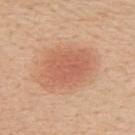Captured during whole-body skin photography for melanoma surveillance; the lesion was not biopsied.
The subject is a female in their 50s.
Measured at roughly 5.5 mm in maximum diameter.
A region of skin cropped from a whole-body photographic capture, roughly 15 mm wide.
The total-body-photography lesion software estimated an area of roughly 17 mm², an eccentricity of roughly 0.75, and two-axis asymmetry of about 0.25. It also reported border irregularity of about 2.5 on a 0–10 scale, internal color variation of about 2.5 on a 0–10 scale, and a peripheral color-asymmetry measure near 0.5.
This is a white-light tile.
Located on the upper back.